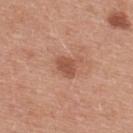Automated image analysis of the tile measured an average lesion color of about L≈52 a*≈25 b*≈32 (CIELAB), a lesion–skin lightness drop of about 10, and a normalized lesion–skin contrast near 7. The analysis additionally found a within-lesion color-variation index near 1/10 and peripheral color asymmetry of about 0.5. The subject is a male aged around 30. The recorded lesion diameter is about 2.5 mm. Located on the upper back. This image is a 15 mm lesion crop taken from a total-body photograph. This is a white-light tile.